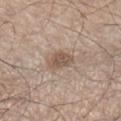The lesion's longest dimension is about 3 mm. Captured under white-light illumination. A 15 mm close-up extracted from a 3D total-body photography capture. From the left lower leg. A male patient aged approximately 45.